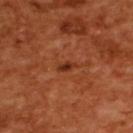follow-up: imaged on a skin check; not biopsied
image source: ~15 mm crop, total-body skin-cancer survey
site: the upper back
subject: female, approximately 55 years of age